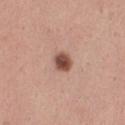| field | value |
|---|---|
| location | the leg |
| tile lighting | white-light |
| image source | total-body-photography crop, ~15 mm field of view |
| lesion diameter | about 2.5 mm |
| patient | female, about 30 years old |
| automated metrics | a lesion color around L≈50 a*≈22 b*≈27 in CIELAB, roughly 16 lightness units darker than nearby skin, and a normalized border contrast of about 11; a nevus-likeness score of about 100/100 |